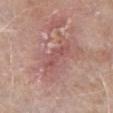From the left forearm. This is a white-light tile. The recorded lesion diameter is about 5 mm. A male patient, aged 73 to 77. A 15 mm crop from a total-body photograph taken for skin-cancer surveillance.